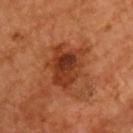notes = imaged on a skin check; not biopsied | subject = male, in their mid-50s | site = the chest | lesion size = ~5.5 mm (longest diameter) | image source = ~15 mm tile from a whole-body skin photo.